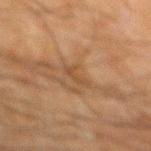biopsy status: catalogued during a skin exam; not biopsied | location: the mid back | patient: male, approximately 65 years of age | image: ~15 mm tile from a whole-body skin photo.